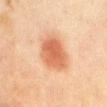The lesion was photographed on a routine skin check and not biopsied; there is no pathology result.
Imaged with cross-polarized lighting.
About 5 mm across.
From the chest.
A female patient roughly 50 years of age.
A 15 mm close-up tile from a total-body photography series done for melanoma screening.